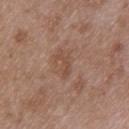<tbp_lesion>
<biopsy_status>not biopsied; imaged during a skin examination</biopsy_status>
<site>upper back</site>
<image>
  <source>total-body photography crop</source>
  <field_of_view_mm>15</field_of_view_mm>
</image>
<patient>
  <sex>male</sex>
  <age_approx>50</age_approx>
</patient>
<lighting>white-light</lighting>
<lesion_size>
  <long_diameter_mm_approx>3.0</long_diameter_mm_approx>
</lesion_size>
</tbp_lesion>